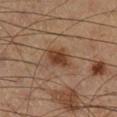notes=catalogued during a skin exam; not biopsied
image source=total-body-photography crop, ~15 mm field of view
body site=the right lower leg
subject=male, in their 60s
illumination=cross-polarized illumination